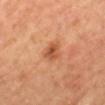The lesion was photographed on a routine skin check and not biopsied; there is no pathology result. The tile uses cross-polarized illumination. A roughly 15 mm field-of-view crop from a total-body skin photograph. The lesion is located on the mid back. The subject is female.